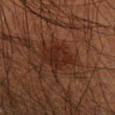Recorded during total-body skin imaging; not selected for excision or biopsy.
The patient is a male aged around 55.
Approximately 4 mm at its widest.
The tile uses cross-polarized illumination.
From the left forearm.
Cropped from a total-body skin-imaging series; the visible field is about 15 mm.